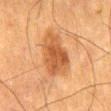The lesion was photographed on a routine skin check and not biopsied; there is no pathology result. A male subject aged 48 to 52. Imaged with cross-polarized lighting. A roughly 15 mm field-of-view crop from a total-body skin photograph. Measured at roughly 5.5 mm in maximum diameter. From the back.